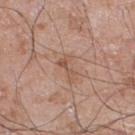Impression: The lesion was tiled from a total-body skin photograph and was not biopsied. Background: Automated image analysis of the tile measured a border-irregularity index near 7.5/10 and radial color variation of about 0. It also reported lesion-presence confidence of about 100/100. From the right lower leg. The patient is a male aged approximately 60. A roughly 15 mm field-of-view crop from a total-body skin photograph. The recorded lesion diameter is about 3 mm. Captured under white-light illumination.